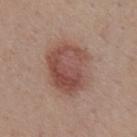Assessment:
This lesion was catalogued during total-body skin photography and was not selected for biopsy.
Background:
Located on the chest. Cropped from a whole-body photographic skin survey; the tile spans about 15 mm. Captured under white-light illumination. A male patient, about 55 years old. The recorded lesion diameter is about 7 mm.